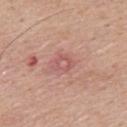Clinical summary:
The tile uses white-light illumination. Located on the upper back. A region of skin cropped from a whole-body photographic capture, roughly 15 mm wide. A male patient about 55 years old.